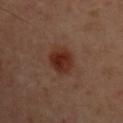The lesion was tiled from a total-body skin photograph and was not biopsied. The patient is a male aged 48 to 52. From the upper back. A 15 mm close-up tile from a total-body photography series done for melanoma screening.